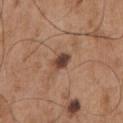Q: How large is the lesion?
A: ≈2.5 mm
Q: Patient demographics?
A: male, in their mid- to late 50s
Q: What is the anatomic site?
A: the chest
Q: What is the imaging modality?
A: ~15 mm crop, total-body skin-cancer survey
Q: What lighting was used for the tile?
A: white-light illumination